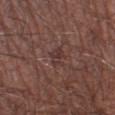{"biopsy_status": "not biopsied; imaged during a skin examination", "image": {"source": "total-body photography crop", "field_of_view_mm": 15}, "lesion_size": {"long_diameter_mm_approx": 3.0}, "automated_metrics": {"border_irregularity_0_10": 4.0, "color_variation_0_10": 2.5, "peripheral_color_asymmetry": 1.0}, "site": "left lower leg", "patient": {"sex": "male", "age_approx": 60}}